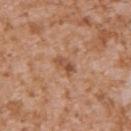{"biopsy_status": "not biopsied; imaged during a skin examination", "patient": {"sex": "male", "age_approx": 45}, "lesion_size": {"long_diameter_mm_approx": 2.5}, "site": "left upper arm", "image": {"source": "total-body photography crop", "field_of_view_mm": 15}, "lighting": "white-light"}